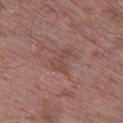Impression:
No biopsy was performed on this lesion — it was imaged during a full skin examination and was not determined to be concerning.
Clinical summary:
From the left thigh. Imaged with white-light lighting. The subject is a male about 50 years old. Measured at roughly 3.5 mm in maximum diameter. This image is a 15 mm lesion crop taken from a total-body photograph.